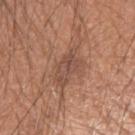Impression:
The lesion was photographed on a routine skin check and not biopsied; there is no pathology result.
Acquisition and patient details:
The lesion is on the right upper arm. A male subject, about 65 years old. About 4.5 mm across. Cropped from a total-body skin-imaging series; the visible field is about 15 mm. Captured under white-light illumination.